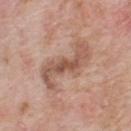The lesion was photographed on a routine skin check and not biopsied; there is no pathology result. A male patient, aged approximately 60. The lesion is on the back. Cropped from a whole-body photographic skin survey; the tile spans about 15 mm.